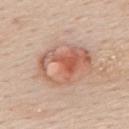biopsy status = no biopsy performed (imaged during a skin exam)
patient = female, approximately 45 years of age
automated lesion analysis = an area of roughly 21 mm², a shape eccentricity near 0.75, and two-axis asymmetry of about 0.3
diameter = ~6.5 mm (longest diameter)
image = total-body-photography crop, ~15 mm field of view
location = the upper back